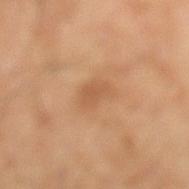Imaged during a routine full-body skin examination; the lesion was not biopsied and no histopathology is available. The lesion is located on the left lower leg. A male patient, in their mid- to late 50s. A 15 mm close-up extracted from a 3D total-body photography capture.